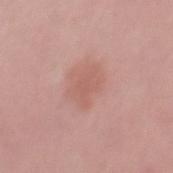Findings:
* workup — total-body-photography surveillance lesion; no biopsy
* tile lighting — white-light
* lesion diameter — about 3.5 mm
* site — the mid back
* patient — male, roughly 30 years of age
* imaging modality — ~15 mm tile from a whole-body skin photo